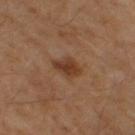A male subject in their mid-50s.
A 15 mm close-up extracted from a 3D total-body photography capture.
On the right thigh.